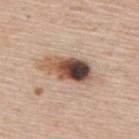Imaged during a routine full-body skin examination; the lesion was not biopsied and no histopathology is available. The lesion is located on the upper back. A region of skin cropped from a whole-body photographic capture, roughly 15 mm wide. A male subject, about 75 years old.